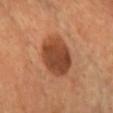biopsy status = catalogued during a skin exam; not biopsied | subject = male, roughly 55 years of age | imaging modality = ~15 mm crop, total-body skin-cancer survey | location = the chest.